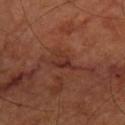notes: no biopsy performed (imaged during a skin exam) | automated metrics: a footprint of about 5 mm² and a symmetry-axis asymmetry near 0.45; an average lesion color of about L≈31 a*≈23 b*≈25 (CIELAB) and a lesion-to-skin contrast of about 6.5 (normalized; higher = more distinct) | size: ~3.5 mm (longest diameter) | subject: in their mid-60s | tile lighting: cross-polarized illumination | location: the leg | acquisition: ~15 mm crop, total-body skin-cancer survey.